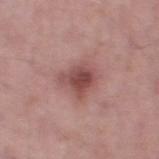<record>
  <biopsy_status>not biopsied; imaged during a skin examination</biopsy_status>
  <site>left thigh</site>
  <lesion_size>
    <long_diameter_mm_approx>3.5</long_diameter_mm_approx>
  </lesion_size>
  <lighting>white-light</lighting>
  <image>
    <source>total-body photography crop</source>
    <field_of_view_mm>15</field_of_view_mm>
  </image>
  <patient>
    <sex>male</sex>
    <age_approx>55</age_approx>
  </patient>
</record>